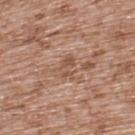<lesion>
<biopsy_status>not biopsied; imaged during a skin examination</biopsy_status>
<image>
  <source>total-body photography crop</source>
  <field_of_view_mm>15</field_of_view_mm>
</image>
<lighting>white-light</lighting>
<patient>
  <sex>male</sex>
  <age_approx>55</age_approx>
</patient>
<site>upper back</site>
</lesion>